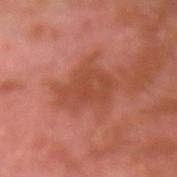follow-up: no biopsy performed (imaged during a skin exam) | diameter: ≈5.5 mm | subject: male, roughly 30 years of age | image source: 15 mm crop, total-body photography | site: the left forearm | TBP lesion metrics: an area of roughly 14 mm², an outline eccentricity of about 0.7 (0 = round, 1 = elongated), and a shape-asymmetry score of about 0.35 (0 = symmetric); a mean CIELAB color near L≈46 a*≈28 b*≈32 and a normalized border contrast of about 6; a within-lesion color-variation index near 2.5/10 and radial color variation of about 1; a nevus-likeness score of about 0/100 | illumination: cross-polarized illumination.